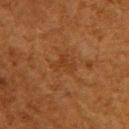Case summary:
* biopsy status · catalogued during a skin exam; not biopsied
* image · 15 mm crop, total-body photography
* diameter · ≈3 mm
* lighting · cross-polarized illumination
* location · the upper back
* subject · female, aged around 50